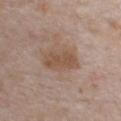Notes:
- notes · total-body-photography surveillance lesion; no biopsy
- anatomic site · the chest
- subject · male, about 80 years old
- image source · total-body-photography crop, ~15 mm field of view
- size · ~4.5 mm (longest diameter)
- illumination · white-light illumination